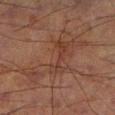Clinical impression:
The lesion was tiled from a total-body skin photograph and was not biopsied.
Acquisition and patient details:
A close-up tile cropped from a whole-body skin photograph, about 15 mm across. A male subject about 70 years old. From the left lower leg.